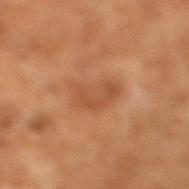The lesion-visualizer software estimated a footprint of about 9.5 mm², an outline eccentricity of about 0.8 (0 = round, 1 = elongated), and a symmetry-axis asymmetry near 0.3. And it measured a border-irregularity rating of about 3/10 and radial color variation of about 1.
The tile uses cross-polarized illumination.
Longest diameter approximately 4.5 mm.
A 15 mm close-up extracted from a 3D total-body photography capture.
A male subject, roughly 65 years of age.